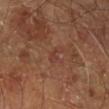Imaged during a routine full-body skin examination; the lesion was not biopsied and no histopathology is available.
A close-up tile cropped from a whole-body skin photograph, about 15 mm across.
The lesion-visualizer software estimated internal color variation of about 0 on a 0–10 scale and radial color variation of about 0. The analysis additionally found a classifier nevus-likeness of about 0/100 and a detector confidence of about 100 out of 100 that the crop contains a lesion.
A male subject, about 60 years old.
The recorded lesion diameter is about 2.5 mm.
The tile uses cross-polarized illumination.
The lesion is on the right leg.